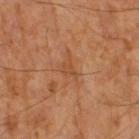<case>
  <biopsy_status>not biopsied; imaged during a skin examination</biopsy_status>
  <image>
    <source>total-body photography crop</source>
    <field_of_view_mm>15</field_of_view_mm>
  </image>
  <patient>
    <sex>male</sex>
    <age_approx>65</age_approx>
  </patient>
  <lesion_size>
    <long_diameter_mm_approx>2.5</long_diameter_mm_approx>
  </lesion_size>
  <lighting>cross-polarized</lighting>
</case>